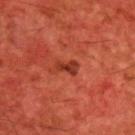workup = catalogued during a skin exam; not biopsied
subject = male, roughly 60 years of age
site = the upper back
illumination = cross-polarized
lesion size = ≈2.5 mm
image-analysis metrics = an average lesion color of about L≈36 a*≈34 b*≈34 (CIELAB) and roughly 10 lightness units darker than nearby skin; border irregularity of about 3.5 on a 0–10 scale, a within-lesion color-variation index near 1/10, and radial color variation of about 0.5; a classifier nevus-likeness of about 35/100 and lesion-presence confidence of about 100/100
image source = 15 mm crop, total-body photography